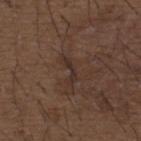{
  "biopsy_status": "not biopsied; imaged during a skin examination",
  "lesion_size": {
    "long_diameter_mm_approx": 3.5
  },
  "patient": {
    "sex": "male",
    "age_approx": 50
  },
  "site": "upper back",
  "image": {
    "source": "total-body photography crop",
    "field_of_view_mm": 15
  }
}